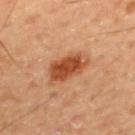Notes:
- biopsy status — no biopsy performed (imaged during a skin exam)
- subject — male, roughly 55 years of age
- anatomic site — the upper back
- tile lighting — cross-polarized illumination
- diameter — ≈5 mm
- imaging modality — ~15 mm tile from a whole-body skin photo
- image-analysis metrics — border irregularity of about 3 on a 0–10 scale, internal color variation of about 3 on a 0–10 scale, and radial color variation of about 1